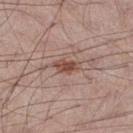Imaged during a routine full-body skin examination; the lesion was not biopsied and no histopathology is available. On the right thigh. The patient is a male aged around 55. The recorded lesion diameter is about 3 mm. Captured under white-light illumination. This image is a 15 mm lesion crop taken from a total-body photograph.